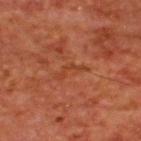follow-up: imaged on a skin check; not biopsied
image source: ~15 mm tile from a whole-body skin photo
subject: male, approximately 65 years of age
diameter: ≈4 mm
automated metrics: a lesion area of about 3.5 mm², an outline eccentricity of about 0.95 (0 = round, 1 = elongated), and a shape-asymmetry score of about 0.75 (0 = symmetric); a border-irregularity rating of about 8.5/10, a color-variation rating of about 0/10, and radial color variation of about 0; an automated nevus-likeness rating near 0 out of 100
location: the upper back
lighting: cross-polarized illumination